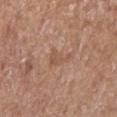| field | value |
|---|---|
| follow-up | imaged on a skin check; not biopsied |
| image | ~15 mm crop, total-body skin-cancer survey |
| subject | female, aged 73–77 |
| body site | the arm |
| illumination | white-light illumination |
| image-analysis metrics | an outline eccentricity of about 0.85 (0 = round, 1 = elongated) and a symmetry-axis asymmetry near 0.5; a mean CIELAB color near L≈53 a*≈20 b*≈29, about 6 CIELAB-L* units darker than the surrounding skin, and a normalized border contrast of about 4.5 |